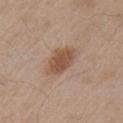biopsy_status: not biopsied; imaged during a skin examination
image:
  source: total-body photography crop
  field_of_view_mm: 15
patient:
  sex: male
  age_approx: 55
site: arm